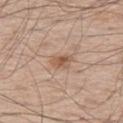Impression:
Part of a total-body skin-imaging series; this lesion was reviewed on a skin check and was not flagged for biopsy.
Clinical summary:
The subject is a male about 60 years old. The tile uses white-light illumination. The lesion is on the left thigh. Automated image analysis of the tile measured an outline eccentricity of about 0.8 (0 = round, 1 = elongated) and a shape-asymmetry score of about 0.25 (0 = symmetric). It also reported a border-irregularity rating of about 2.5/10, internal color variation of about 3.5 on a 0–10 scale, and peripheral color asymmetry of about 1. It also reported an automated nevus-likeness rating near 70 out of 100 and lesion-presence confidence of about 100/100. Longest diameter approximately 3 mm. A roughly 15 mm field-of-view crop from a total-body skin photograph.